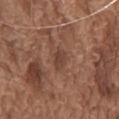Recorded during total-body skin imaging; not selected for excision or biopsy. Approximately 2.5 mm at its widest. Automated image analysis of the tile measured a shape eccentricity near 0.85 and a shape-asymmetry score of about 0.3 (0 = symmetric). And it measured a border-irregularity index near 2.5/10, internal color variation of about 1 on a 0–10 scale, and radial color variation of about 0.5. The analysis additionally found an automated nevus-likeness rating near 10 out of 100 and a detector confidence of about 100 out of 100 that the crop contains a lesion. A male patient, aged around 75. The lesion is located on the chest. Captured under white-light illumination. Cropped from a total-body skin-imaging series; the visible field is about 15 mm.